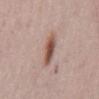lighting: white-light; lesion size: ~4 mm (longest diameter); subject: male, in their 50s; image source: ~15 mm crop, total-body skin-cancer survey; location: the mid back.